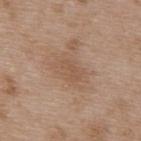Captured during whole-body skin photography for melanoma surveillance; the lesion was not biopsied. On the back. A 15 mm crop from a total-body photograph taken for skin-cancer surveillance. A male subject approximately 50 years of age.